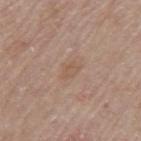Q: What are the patient's age and sex?
A: male, aged 78 to 82
Q: What kind of image is this?
A: ~15 mm tile from a whole-body skin photo
Q: Where on the body is the lesion?
A: the back